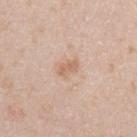workup: total-body-photography surveillance lesion; no biopsy
lesion diameter: ~2.5 mm (longest diameter)
image: total-body-photography crop, ~15 mm field of view
lighting: white-light illumination
patient: male, approximately 40 years of age
body site: the right upper arm